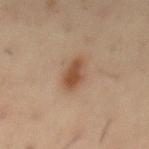Captured during whole-body skin photography for melanoma surveillance; the lesion was not biopsied. A male subject, aged 53–57. From the mid back. This image is a 15 mm lesion crop taken from a total-body photograph.